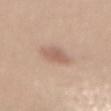biopsy_status: not biopsied; imaged during a skin examination
automated_metrics:
  area_mm2_approx: 5.5
  shape_asymmetry: 0.3
  cielab_L: 59
  cielab_a: 19
  cielab_b: 27
  vs_skin_darker_L: 9.0
  vs_skin_contrast_norm: 6.0
patient:
  sex: female
  age_approx: 35
lesion_size:
  long_diameter_mm_approx: 3.0
image:
  source: total-body photography crop
  field_of_view_mm: 15
site: mid back
lighting: white-light